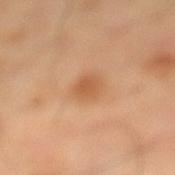Assessment:
The lesion was photographed on a routine skin check and not biopsied; there is no pathology result.
Image and clinical context:
The lesion's longest dimension is about 2.5 mm. A female subject roughly 60 years of age. Captured under cross-polarized illumination. Automated tile analysis of the lesion measured an average lesion color of about L≈58 a*≈24 b*≈39 (CIELAB), a lesion–skin lightness drop of about 9, and a normalized border contrast of about 6.5. The software also gave a border-irregularity rating of about 2/10, internal color variation of about 1.5 on a 0–10 scale, and a peripheral color-asymmetry measure near 0.5. It also reported a nevus-likeness score of about 60/100 and a detector confidence of about 100 out of 100 that the crop contains a lesion. On the right leg. A lesion tile, about 15 mm wide, cut from a 3D total-body photograph.